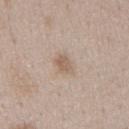Part of a total-body skin-imaging series; this lesion was reviewed on a skin check and was not flagged for biopsy.
Cropped from a total-body skin-imaging series; the visible field is about 15 mm.
The recorded lesion diameter is about 3 mm.
The patient is a male in their 50s.
The total-body-photography lesion software estimated a mean CIELAB color near L≈59 a*≈15 b*≈27, about 9 CIELAB-L* units darker than the surrounding skin, and a normalized lesion–skin contrast near 6.5.
Located on the mid back.
The tile uses white-light illumination.